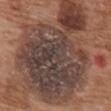notes: total-body-photography surveillance lesion; no biopsy
location: the mid back
TBP lesion metrics: a footprint of about 100 mm², an eccentricity of roughly 0.55, and two-axis asymmetry of about 0.35; an average lesion color of about L≈41 a*≈16 b*≈22 (CIELAB), a lesion–skin lightness drop of about 12, and a normalized lesion–skin contrast near 11
patient: female, roughly 75 years of age
lesion size: ≈13.5 mm
tile lighting: white-light
acquisition: total-body-photography crop, ~15 mm field of view